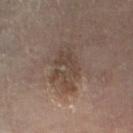• workup — total-body-photography surveillance lesion; no biopsy
• site — the left thigh
• image source — ~15 mm crop, total-body skin-cancer survey
• TBP lesion metrics — a footprint of about 13 mm², an eccentricity of roughly 0.85, and a symmetry-axis asymmetry near 0.3; a border-irregularity rating of about 4.5/10 and radial color variation of about 1
• lesion size — about 6 mm
• patient — female, aged around 60
• tile lighting — cross-polarized illumination